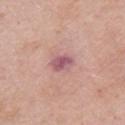Impression:
Captured during whole-body skin photography for melanoma surveillance; the lesion was not biopsied.
Image and clinical context:
From the right upper arm. A close-up tile cropped from a whole-body skin photograph, about 15 mm across. A female subject, approximately 70 years of age.